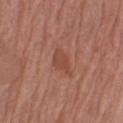Assessment:
Captured during whole-body skin photography for melanoma surveillance; the lesion was not biopsied.
Context:
Imaged with white-light lighting. On the right upper arm. Longest diameter approximately 3.5 mm. A male patient, aged around 75. A 15 mm close-up tile from a total-body photography series done for melanoma screening.